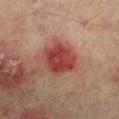| feature | finding |
|---|---|
| follow-up | catalogued during a skin exam; not biopsied |
| size | about 5 mm |
| image source | total-body-photography crop, ~15 mm field of view |
| site | the right lower leg |
| subject | male, approximately 75 years of age |